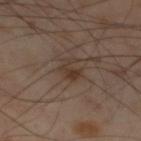A roughly 15 mm field-of-view crop from a total-body skin photograph. The lesion-visualizer software estimated a footprint of about 4.5 mm², a shape eccentricity near 0.45, and a shape-asymmetry score of about 0.35 (0 = symmetric). It also reported a detector confidence of about 100 out of 100 that the crop contains a lesion. A male subject, aged approximately 55. The tile uses cross-polarized illumination. The lesion is on the left lower leg.